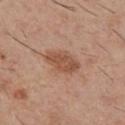{"biopsy_status": "not biopsied; imaged during a skin examination", "patient": {"sex": "male", "age_approx": 30}, "site": "chest", "image": {"source": "total-body photography crop", "field_of_view_mm": 15}, "lighting": "white-light", "lesion_size": {"long_diameter_mm_approx": 4.0}}